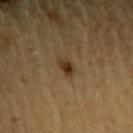Clinical impression:
Imaged during a routine full-body skin examination; the lesion was not biopsied and no histopathology is available.
Background:
From the left upper arm. A male patient, roughly 85 years of age or older. This image is a 15 mm lesion crop taken from a total-body photograph.